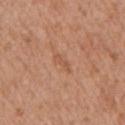biopsy_status: not biopsied; imaged during a skin examination
lesion_size:
  long_diameter_mm_approx: 3.0
lighting: white-light
site: arm
patient:
  sex: female
  age_approx: 75
image:
  source: total-body photography crop
  field_of_view_mm: 15